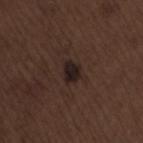Assessment:
No biopsy was performed on this lesion — it was imaged during a full skin examination and was not determined to be concerning.
Clinical summary:
The lesion-visualizer software estimated a mean CIELAB color near L≈20 a*≈12 b*≈14, a lesion–skin lightness drop of about 8, and a normalized border contrast of about 11.5. And it measured a border-irregularity rating of about 2.5/10 and peripheral color asymmetry of about 0.5. The recorded lesion diameter is about 3 mm. A male subject about 70 years old. The tile uses white-light illumination. Cropped from a whole-body photographic skin survey; the tile spans about 15 mm. From the lower back.